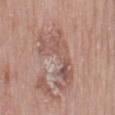Case summary:
• notes: total-body-photography surveillance lesion; no biopsy